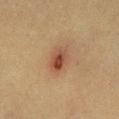<case>
<biopsy_status>not biopsied; imaged during a skin examination</biopsy_status>
<image>
  <source>total-body photography crop</source>
  <field_of_view_mm>15</field_of_view_mm>
</image>
<site>left lower leg</site>
<patient>
  <sex>female</sex>
  <age_approx>55</age_approx>
</patient>
<lesion_size>
  <long_diameter_mm_approx>3.0</long_diameter_mm_approx>
</lesion_size>
</case>